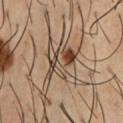Notes:
– follow-up · total-body-photography surveillance lesion; no biopsy
– patient · male, in their mid- to late 50s
– site · the chest
– diameter · about 4 mm
– imaging modality · 15 mm crop, total-body photography